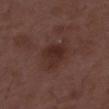Notes:
• follow-up: catalogued during a skin exam; not biopsied
• tile lighting: white-light illumination
• acquisition: 15 mm crop, total-body photography
• TBP lesion metrics: an eccentricity of roughly 0.5; a mean CIELAB color near L≈28 a*≈19 b*≈21, about 7 CIELAB-L* units darker than the surrounding skin, and a normalized lesion–skin contrast near 7.5
• subject: male, aged approximately 50